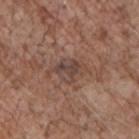  biopsy_status: not biopsied; imaged during a skin examination
  patient:
    sex: male
    age_approx: 70
  site: chest
  lighting: white-light
  automated_metrics:
    color_variation_0_10: 5.5
    peripheral_color_asymmetry: 2.0
  image:
    source: total-body photography crop
    field_of_view_mm: 15
  lesion_size:
    long_diameter_mm_approx: 4.0